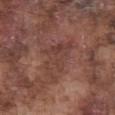biopsy status = imaged on a skin check; not biopsied
lesion size = ≈5.5 mm
image source = total-body-photography crop, ~15 mm field of view
subject = male, about 75 years old
location = the front of the torso